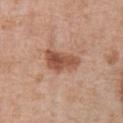{
  "biopsy_status": "not biopsied; imaged during a skin examination",
  "lighting": "white-light",
  "automated_metrics": {
    "area_mm2_approx": 8.5,
    "shape_asymmetry": 0.35,
    "border_irregularity_0_10": 3.5,
    "color_variation_0_10": 4.5,
    "peripheral_color_asymmetry": 1.5
  },
  "patient": {
    "sex": "male",
    "age_approx": 70
  },
  "site": "abdomen",
  "image": {
    "source": "total-body photography crop",
    "field_of_view_mm": 15
  },
  "lesion_size": {
    "long_diameter_mm_approx": 4.5
  }
}